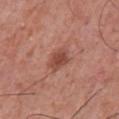Captured during whole-body skin photography for melanoma surveillance; the lesion was not biopsied. A region of skin cropped from a whole-body photographic capture, roughly 15 mm wide. The lesion is on the front of the torso. A male patient aged around 55.